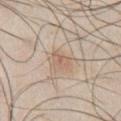Clinical impression:
The lesion was tiled from a total-body skin photograph and was not biopsied.
Acquisition and patient details:
The recorded lesion diameter is about 2.5 mm. A male patient, roughly 45 years of age. Imaged with white-light lighting. On the chest. A 15 mm crop from a total-body photograph taken for skin-cancer surveillance.